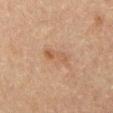acquisition = 15 mm crop, total-body photography; diameter = about 3.5 mm; patient = male, approximately 75 years of age; body site = the front of the torso.